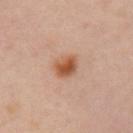follow-up: catalogued during a skin exam; not biopsied | lesion diameter: ~3.5 mm (longest diameter) | site: the left upper arm | subject: female, roughly 40 years of age | illumination: cross-polarized illumination | image: ~15 mm crop, total-body skin-cancer survey | TBP lesion metrics: a lesion area of about 6.5 mm², an eccentricity of roughly 0.75, and a symmetry-axis asymmetry near 0.25; a mean CIELAB color near L≈55 a*≈23 b*≈34 and a normalized lesion–skin contrast near 9; a detector confidence of about 100 out of 100 that the crop contains a lesion.